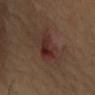Case summary:
• notes: imaged on a skin check; not biopsied
• diameter: about 5.5 mm
• subject: female, roughly 40 years of age
• imaging modality: 15 mm crop, total-body photography
• body site: the chest